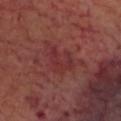Imaged during a routine full-body skin examination; the lesion was not biopsied and no histopathology is available. A male subject approximately 60 years of age. From the head or neck. The total-body-photography lesion software estimated a symmetry-axis asymmetry near 0.5. A region of skin cropped from a whole-body photographic capture, roughly 15 mm wide.